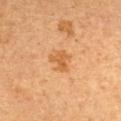{
  "biopsy_status": "not biopsied; imaged during a skin examination",
  "site": "chest",
  "automated_metrics": {
    "area_mm2_approx": 5.5,
    "eccentricity": 0.6,
    "shape_asymmetry": 0.3,
    "cielab_L": 56,
    "cielab_a": 23,
    "cielab_b": 41,
    "vs_skin_darker_L": 8.0,
    "vs_skin_contrast_norm": 6.5,
    "nevus_likeness_0_100": 25,
    "lesion_detection_confidence_0_100": 100
  },
  "image": {
    "source": "total-body photography crop",
    "field_of_view_mm": 15
  },
  "patient": {
    "sex": "female",
    "age_approx": 30
  },
  "lesion_size": {
    "long_diameter_mm_approx": 3.0
  }
}